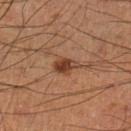follow-up = catalogued during a skin exam; not biopsied | acquisition = ~15 mm crop, total-body skin-cancer survey | diameter = about 3 mm | anatomic site = the leg | subject = male, aged 58–62 | illumination = cross-polarized | automated metrics = border irregularity of about 2 on a 0–10 scale, a color-variation rating of about 3.5/10, and a peripheral color-asymmetry measure near 1.